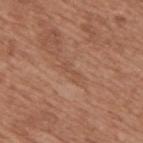No biopsy was performed on this lesion — it was imaged during a full skin examination and was not determined to be concerning. The tile uses white-light illumination. A male subject, roughly 65 years of age. A 15 mm close-up tile from a total-body photography series done for melanoma screening. From the upper back. The total-body-photography lesion software estimated an outline eccentricity of about 0.95 (0 = round, 1 = elongated). The analysis additionally found a border-irregularity rating of about 6.5/10, a within-lesion color-variation index near 0/10, and radial color variation of about 0.